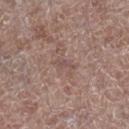Clinical impression: The lesion was tiled from a total-body skin photograph and was not biopsied. Background: A lesion tile, about 15 mm wide, cut from a 3D total-body photograph. The lesion is on the right lower leg. The patient is a male aged 68–72.